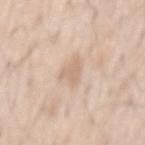– biopsy status — no biopsy performed (imaged during a skin exam)
– automated metrics — a classifier nevus-likeness of about 0/100 and a detector confidence of about 100 out of 100 that the crop contains a lesion
– acquisition — ~15 mm crop, total-body skin-cancer survey
– patient — male, about 60 years old
– lesion size — ~3 mm (longest diameter)
– lighting — white-light illumination
– location — the mid back